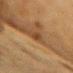- notes · imaged on a skin check; not biopsied
- anatomic site · the chest
- lighting · cross-polarized
- lesion diameter · ~2.5 mm (longest diameter)
- automated lesion analysis · a footprint of about 3 mm² and an outline eccentricity of about 0.8 (0 = round, 1 = elongated); a nevus-likeness score of about 0/100 and a detector confidence of about 60 out of 100 that the crop contains a lesion
- acquisition · total-body-photography crop, ~15 mm field of view
- subject · female, aged approximately 55